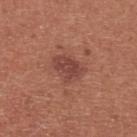Impression: This lesion was catalogued during total-body skin photography and was not selected for biopsy.